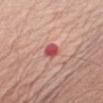<case>
  <biopsy_status>not biopsied; imaged during a skin examination</biopsy_status>
  <image>
    <source>total-body photography crop</source>
    <field_of_view_mm>15</field_of_view_mm>
  </image>
  <lighting>white-light</lighting>
  <lesion_size>
    <long_diameter_mm_approx>2.5</long_diameter_mm_approx>
  </lesion_size>
  <patient>
    <sex>male</sex>
    <age_approx>60</age_approx>
  </patient>
  <site>right upper arm</site>
  <automated_metrics>
    <eccentricity>0.7</eccentricity>
    <shape_asymmetry>0.2</shape_asymmetry>
    <cielab_L>53</cielab_L>
    <cielab_a>35</cielab_a>
    <cielab_b>24</cielab_b>
    <vs_skin_darker_L>14.0</vs_skin_darker_L>
    <vs_skin_contrast_norm>9.0</vs_skin_contrast_norm>
    <border_irregularity_0_10>2.0</border_irregularity_0_10>
    <color_variation_0_10>4.0</color_variation_0_10>
    <peripheral_color_asymmetry>1.5</peripheral_color_asymmetry>
    <nevus_likeness_0_100>0</nevus_likeness_0_100>
    <lesion_detection_confidence_0_100>100</lesion_detection_confidence_0_100>
  </automated_metrics>
</case>